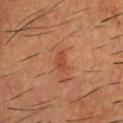Clinical impression:
The lesion was tiled from a total-body skin photograph and was not biopsied.
Acquisition and patient details:
Automated image analysis of the tile measured a normalized lesion–skin contrast near 6. The analysis additionally found a nevus-likeness score of about 5/100 and a lesion-detection confidence of about 100/100. This image is a 15 mm lesion crop taken from a total-body photograph. The lesion is on the upper back. A male subject, approximately 55 years of age.